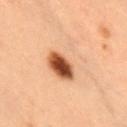Notes:
– follow-up — imaged on a skin check; not biopsied
– lighting — cross-polarized
– image — total-body-photography crop, ~15 mm field of view
– lesion size — about 5.5 mm
– location — the chest
– patient — female, aged approximately 40